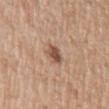Assessment:
No biopsy was performed on this lesion — it was imaged during a full skin examination and was not determined to be concerning.
Acquisition and patient details:
The lesion is on the arm. The tile uses white-light illumination. Cropped from a total-body skin-imaging series; the visible field is about 15 mm. A male patient about 60 years old.